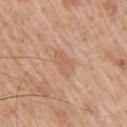Imaged during a routine full-body skin examination; the lesion was not biopsied and no histopathology is available. The lesion is located on the right upper arm. Captured under white-light illumination. Longest diameter approximately 3.5 mm. The lesion-visualizer software estimated a lesion area of about 5.5 mm² and two-axis asymmetry of about 0.25. The analysis additionally found a within-lesion color-variation index near 2/10 and a peripheral color-asymmetry measure near 0.5. It also reported an automated nevus-likeness rating near 0 out of 100 and a detector confidence of about 100 out of 100 that the crop contains a lesion. A 15 mm close-up tile from a total-body photography series done for melanoma screening. A male patient, aged 58–62.